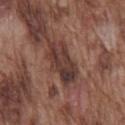Notes:
- site — the chest
- acquisition — ~15 mm tile from a whole-body skin photo
- lesion diameter — about 5.5 mm
- lighting — white-light
- image-analysis metrics — a footprint of about 14 mm² and an eccentricity of roughly 0.85; an automated nevus-likeness rating near 0 out of 100 and a lesion-detection confidence of about 65/100
- patient — male, roughly 75 years of age